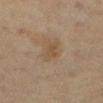Recorded during total-body skin imaging; not selected for excision or biopsy. The tile uses cross-polarized illumination. The patient is a female approximately 50 years of age. On the left lower leg. Approximately 3 mm at its widest. Cropped from a whole-body photographic skin survey; the tile spans about 15 mm.